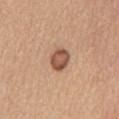| field | value |
|---|---|
| subject | female, aged 53–57 |
| lighting | white-light illumination |
| automated metrics | about 14 CIELAB-L* units darker than the surrounding skin; border irregularity of about 1 on a 0–10 scale, a color-variation rating of about 3/10, and a peripheral color-asymmetry measure near 1; an automated nevus-likeness rating near 25 out of 100 |
| body site | the mid back |
| diameter | ~3 mm (longest diameter) |
| image source | 15 mm crop, total-body photography |